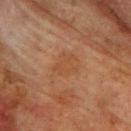No biopsy was performed on this lesion — it was imaged during a full skin examination and was not determined to be concerning.
The patient is a male aged around 75.
A region of skin cropped from a whole-body photographic capture, roughly 15 mm wide.
On the back.